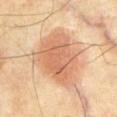{"biopsy_status": "not biopsied; imaged during a skin examination", "patient": {"sex": "male", "age_approx": 70}, "lighting": "cross-polarized", "lesion_size": {"long_diameter_mm_approx": 8.0}, "image": {"source": "total-body photography crop", "field_of_view_mm": 15}, "site": "chest", "automated_metrics": {"cielab_L": 66, "cielab_a": 22, "cielab_b": 35, "vs_skin_darker_L": 12.0, "vs_skin_contrast_norm": 7.0, "border_irregularity_0_10": 3.5, "color_variation_0_10": 4.0, "peripheral_color_asymmetry": 1.0}}